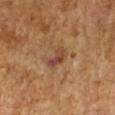A female subject approximately 70 years of age.
The tile uses cross-polarized illumination.
The lesion is on the right forearm.
A close-up tile cropped from a whole-body skin photograph, about 15 mm across.
Automated image analysis of the tile measured border irregularity of about 4.5 on a 0–10 scale, a color-variation rating of about 9.5/10, and peripheral color asymmetry of about 3.5.
Longest diameter approximately 3 mm.